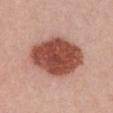follow-up: total-body-photography surveillance lesion; no biopsy
image source: 15 mm crop, total-body photography
location: the chest
tile lighting: white-light illumination
automated metrics: a shape-asymmetry score of about 0.1 (0 = symmetric); an average lesion color of about L≈49 a*≈27 b*≈28 (CIELAB), roughly 19 lightness units darker than nearby skin, and a normalized lesion–skin contrast near 12.5; a border-irregularity rating of about 1/10, a color-variation rating of about 4/10, and a peripheral color-asymmetry measure near 1.5
lesion diameter: ≈7 mm
subject: female, aged approximately 35